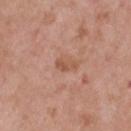Assessment: No biopsy was performed on this lesion — it was imaged during a full skin examination and was not determined to be concerning. Image and clinical context: The subject is a male aged 48 to 52. On the back. Cropped from a total-body skin-imaging series; the visible field is about 15 mm. Measured at roughly 3 mm in maximum diameter. This is a white-light tile. Automated image analysis of the tile measured an eccentricity of roughly 0.9 and two-axis asymmetry of about 0.45. The software also gave an average lesion color of about L≈54 a*≈23 b*≈31 (CIELAB), about 8 CIELAB-L* units darker than the surrounding skin, and a normalized lesion–skin contrast near 6. The software also gave a classifier nevus-likeness of about 0/100 and a lesion-detection confidence of about 100/100.